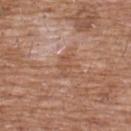Impression:
This lesion was catalogued during total-body skin photography and was not selected for biopsy.
Clinical summary:
Captured under white-light illumination. A male subject about 60 years old. Approximately 3 mm at its widest. On the front of the torso. A 15 mm close-up tile from a total-body photography series done for melanoma screening.